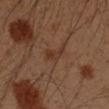The lesion was photographed on a routine skin check and not biopsied; there is no pathology result.
A male patient approximately 50 years of age.
Located on the left upper arm.
A close-up tile cropped from a whole-body skin photograph, about 15 mm across.
Imaged with cross-polarized lighting.